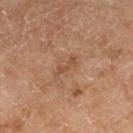No biopsy was performed on this lesion — it was imaged during a full skin examination and was not determined to be concerning. On the leg. A male subject, in their mid- to late 60s. This is a cross-polarized tile. Cropped from a whole-body photographic skin survey; the tile spans about 15 mm. The lesion-visualizer software estimated an area of roughly 3.5 mm² and an eccentricity of roughly 0.95. It also reported a lesion color around L≈50 a*≈21 b*≈33 in CIELAB, roughly 7 lightness units darker than nearby skin, and a lesion-to-skin contrast of about 5.5 (normalized; higher = more distinct). It also reported an automated nevus-likeness rating near 0 out of 100. The recorded lesion diameter is about 3.5 mm.